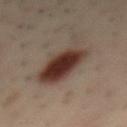The lesion was tiled from a total-body skin photograph and was not biopsied.
The tile uses cross-polarized illumination.
A 15 mm close-up extracted from a 3D total-body photography capture.
Automated tile analysis of the lesion measured a footprint of about 19 mm², an eccentricity of roughly 0.85, and two-axis asymmetry of about 0.3. It also reported a border-irregularity index near 4/10, a within-lesion color-variation index near 7.5/10, and radial color variation of about 2.
A male subject roughly 40 years of age.
The recorded lesion diameter is about 7.5 mm.
On the mid back.